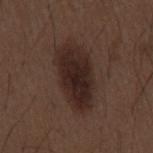Part of a total-body skin-imaging series; this lesion was reviewed on a skin check and was not flagged for biopsy.
Automated image analysis of the tile measured a shape eccentricity near 0.85 and two-axis asymmetry of about 0.15. The analysis additionally found a classifier nevus-likeness of about 85/100 and lesion-presence confidence of about 100/100.
The tile uses white-light illumination.
A lesion tile, about 15 mm wide, cut from a 3D total-body photograph.
The subject is a male aged 48–52.
On the mid back.